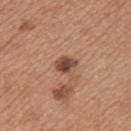biopsy_status: not biopsied; imaged during a skin examination
image:
  source: total-body photography crop
  field_of_view_mm: 15
lighting: white-light
patient:
  sex: female
  age_approx: 35
automated_metrics:
  area_mm2_approx: 4.5
  eccentricity: 0.6
  cielab_L: 47
  cielab_a: 23
  cielab_b: 28
  vs_skin_contrast_norm: 10.0
  border_irregularity_0_10: 2.0
  peripheral_color_asymmetry: 1.5
site: left upper arm